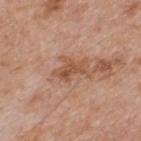Captured during whole-body skin photography for melanoma surveillance; the lesion was not biopsied.
This is a white-light tile.
The patient is a male aged approximately 60.
From the upper back.
Cropped from a whole-body photographic skin survey; the tile spans about 15 mm.
Measured at roughly 3.5 mm in maximum diameter.
Automated tile analysis of the lesion measured an average lesion color of about L≈52 a*≈23 b*≈32 (CIELAB), roughly 10 lightness units darker than nearby skin, and a normalized border contrast of about 7. The software also gave border irregularity of about 4 on a 0–10 scale, a color-variation rating of about 3/10, and peripheral color asymmetry of about 1. The analysis additionally found a detector confidence of about 100 out of 100 that the crop contains a lesion.